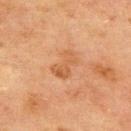Recorded during total-body skin imaging; not selected for excision or biopsy.
The lesion is located on the back.
A male subject aged 68–72.
A lesion tile, about 15 mm wide, cut from a 3D total-body photograph.
Imaged with cross-polarized lighting.
Automated tile analysis of the lesion measured a lesion–skin lightness drop of about 7. The analysis additionally found a nevus-likeness score of about 0/100.
About 3.5 mm across.